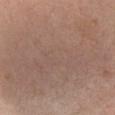patient = female, in their 40s | image source = ~15 mm crop, total-body skin-cancer survey | size = about 17.5 mm | location = the chest | illumination = white-light | TBP lesion metrics = a border-irregularity index near 3/10, internal color variation of about 4 on a 0–10 scale, and radial color variation of about 1; lesion-presence confidence of about 75/100.